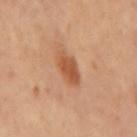Notes:
• size — about 4.5 mm
• location — the mid back
• automated metrics — a lesion color around L≈55 a*≈25 b*≈37 in CIELAB, a lesion–skin lightness drop of about 11, and a lesion-to-skin contrast of about 7.5 (normalized; higher = more distinct); a border-irregularity index near 2.5/10, a color-variation rating of about 3/10, and radial color variation of about 1
• imaging modality — total-body-photography crop, ~15 mm field of view
• tile lighting — cross-polarized